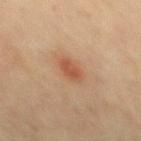No biopsy was performed on this lesion — it was imaged during a full skin examination and was not determined to be concerning. From the mid back. This is a cross-polarized tile. Automated tile analysis of the lesion measured a lesion area of about 4 mm², an outline eccentricity of about 0.85 (0 = round, 1 = elongated), and a symmetry-axis asymmetry near 0.25. And it measured border irregularity of about 2.5 on a 0–10 scale, a color-variation rating of about 1/10, and a peripheral color-asymmetry measure near 0.5. The analysis additionally found an automated nevus-likeness rating near 100 out of 100 and a detector confidence of about 100 out of 100 that the crop contains a lesion. A 15 mm crop from a total-body photograph taken for skin-cancer surveillance. The patient is a male in their mid-40s. The recorded lesion diameter is about 3 mm.